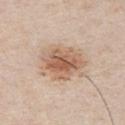<case>
<lesion_size>
  <long_diameter_mm_approx>5.0</long_diameter_mm_approx>
</lesion_size>
<patient>
  <sex>male</sex>
  <age_approx>50</age_approx>
</patient>
<lighting>white-light</lighting>
<site>chest</site>
<image>
  <source>total-body photography crop</source>
  <field_of_view_mm>15</field_of_view_mm>
</image>
</case>